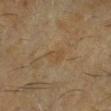Recorded during total-body skin imaging; not selected for excision or biopsy. On the right lower leg. This image is a 15 mm lesion crop taken from a total-body photograph. The lesion's longest dimension is about 2.5 mm. A male subject, aged approximately 60. Imaged with cross-polarized lighting.